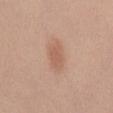| key | value |
|---|---|
| notes | catalogued during a skin exam; not biopsied |
| lighting | white-light |
| TBP lesion metrics | a shape eccentricity near 0.85 and two-axis asymmetry of about 0.2; a normalized lesion–skin contrast near 5.5; an automated nevus-likeness rating near 85 out of 100 and a lesion-detection confidence of about 100/100 |
| patient | female, roughly 40 years of age |
| location | the mid back |
| image source | 15 mm crop, total-body photography |